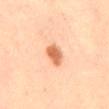notes = total-body-photography surveillance lesion; no biopsy